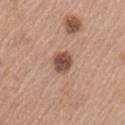Findings:
- workup · total-body-photography surveillance lesion; no biopsy
- lesion diameter · ≈3 mm
- lighting · white-light
- automated metrics · a lesion color around L≈50 a*≈20 b*≈26 in CIELAB, about 14 CIELAB-L* units darker than the surrounding skin, and a normalized lesion–skin contrast near 10; an automated nevus-likeness rating near 75 out of 100 and a lesion-detection confidence of about 100/100
- site · the left upper arm
- patient · female, roughly 65 years of age
- image source · ~15 mm tile from a whole-body skin photo